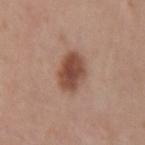biopsy_status: not biopsied; imaged during a skin examination
image:
  source: total-body photography crop
  field_of_view_mm: 15
patient:
  sex: female
  age_approx: 35
site: arm
lesion_size:
  long_diameter_mm_approx: 4.0
lighting: white-light